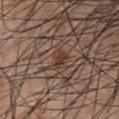Q: What is the imaging modality?
A: total-body-photography crop, ~15 mm field of view
Q: Lesion location?
A: the chest
Q: What is the lesion's diameter?
A: ≈3 mm
Q: How was the tile lit?
A: white-light
Q: Patient demographics?
A: male, aged around 55